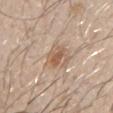Captured during whole-body skin photography for melanoma surveillance; the lesion was not biopsied.
The tile uses white-light illumination.
The lesion is on the right upper arm.
A roughly 15 mm field-of-view crop from a total-body skin photograph.
About 2.5 mm across.
A male subject, aged around 30.